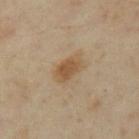Imaged during a routine full-body skin examination; the lesion was not biopsied and no histopathology is available. A roughly 15 mm field-of-view crop from a total-body skin photograph. Located on the right thigh. The subject is a female roughly 35 years of age.